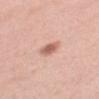Notes:
- follow-up: total-body-photography surveillance lesion; no biopsy
- lighting: white-light
- lesion diameter: ~2.5 mm (longest diameter)
- site: the upper back
- patient: female, about 40 years old
- image: 15 mm crop, total-body photography